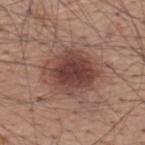biopsy status: no biopsy performed (imaged during a skin exam)
tile lighting: white-light
patient: male, roughly 60 years of age
imaging modality: 15 mm crop, total-body photography
anatomic site: the upper back
lesion size: ≈6 mm
automated metrics: a footprint of about 18 mm², an outline eccentricity of about 0.6 (0 = round, 1 = elongated), and a shape-asymmetry score of about 0.2 (0 = symmetric); a mean CIELAB color near L≈42 a*≈22 b*≈23, a lesion–skin lightness drop of about 13, and a normalized lesion–skin contrast near 10; an automated nevus-likeness rating near 95 out of 100